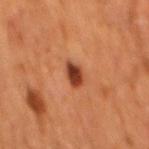Q: What are the patient's age and sex?
A: male, roughly 65 years of age
Q: What is the anatomic site?
A: the back
Q: How was the tile lit?
A: cross-polarized
Q: What is the lesion's diameter?
A: ≈3 mm
Q: What did automated image analysis measure?
A: border irregularity of about 2.5 on a 0–10 scale, a color-variation rating of about 4/10, and radial color variation of about 1
Q: How was this image acquired?
A: ~15 mm crop, total-body skin-cancer survey